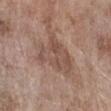The lesion was tiled from a total-body skin photograph and was not biopsied. The total-body-photography lesion software estimated a lesion area of about 16 mm² and an eccentricity of roughly 0.75. The lesion's longest dimension is about 6 mm. A 15 mm close-up tile from a total-body photography series done for melanoma screening. A female subject, aged 73–77. The tile uses white-light illumination. On the right forearm.